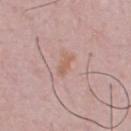Impression:
No biopsy was performed on this lesion — it was imaged during a full skin examination and was not determined to be concerning.
Acquisition and patient details:
A region of skin cropped from a whole-body photographic capture, roughly 15 mm wide. Approximately 3 mm at its widest. Imaged with white-light lighting. A male subject, approximately 50 years of age. Automated image analysis of the tile measured a lesion area of about 3 mm² and a symmetry-axis asymmetry near 0.3. The software also gave a border-irregularity index near 3.5/10, a color-variation rating of about 0.5/10, and a peripheral color-asymmetry measure near 0.5. The lesion is on the chest.